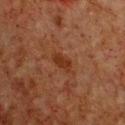Clinical impression: The lesion was photographed on a routine skin check and not biopsied; there is no pathology result. Image and clinical context: Captured under cross-polarized illumination. A male patient, aged 63–67. The lesion is located on the chest. The recorded lesion diameter is about 3 mm. This image is a 15 mm lesion crop taken from a total-body photograph.